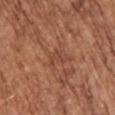The lesion was photographed on a routine skin check and not biopsied; there is no pathology result.
Cropped from a whole-body photographic skin survey; the tile spans about 15 mm.
A female patient aged 73–77.
The total-body-photography lesion software estimated an area of roughly 4 mm² and a shape-asymmetry score of about 0.45 (0 = symmetric).
Located on the arm.
Imaged with white-light lighting.
The recorded lesion diameter is about 3 mm.